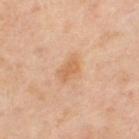{"biopsy_status": "not biopsied; imaged during a skin examination", "patient": {"sex": "female", "age_approx": 50}, "image": {"source": "total-body photography crop", "field_of_view_mm": 15}, "site": "right upper arm"}